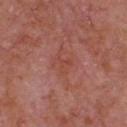biopsy status: total-body-photography surveillance lesion; no biopsy
automated metrics: a footprint of about 3 mm², an outline eccentricity of about 0.65 (0 = round, 1 = elongated), and a symmetry-axis asymmetry near 0.55; an average lesion color of about L≈46 a*≈28 b*≈29 (CIELAB), roughly 5 lightness units darker than nearby skin, and a normalized lesion–skin contrast near 5; border irregularity of about 6 on a 0–10 scale and a color-variation rating of about 0/10; an automated nevus-likeness rating near 0 out of 100 and a lesion-detection confidence of about 100/100
anatomic site: the front of the torso
lesion diameter: about 2.5 mm
lighting: white-light
image source: 15 mm crop, total-body photography
subject: male, roughly 65 years of age